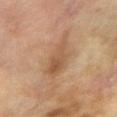Part of a total-body skin-imaging series; this lesion was reviewed on a skin check and was not flagged for biopsy.
The lesion-visualizer software estimated a lesion area of about 11 mm², an eccentricity of roughly 0.85, and two-axis asymmetry of about 0.25. The software also gave an average lesion color of about L≈49 a*≈17 b*≈31 (CIELAB), a lesion–skin lightness drop of about 8, and a lesion-to-skin contrast of about 6 (normalized; higher = more distinct). And it measured border irregularity of about 3.5 on a 0–10 scale, a within-lesion color-variation index near 4.5/10, and radial color variation of about 1.5. The analysis additionally found an automated nevus-likeness rating near 5 out of 100 and lesion-presence confidence of about 100/100.
A 15 mm crop from a total-body photograph taken for skin-cancer surveillance.
Measured at roughly 5.5 mm in maximum diameter.
The lesion is located on the right forearm.
A female patient in their 60s.
This is a cross-polarized tile.